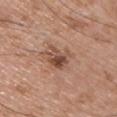The subject is a male aged 48 to 52. The lesion is located on the chest. The lesion's longest dimension is about 3 mm. This image is a 15 mm lesion crop taken from a total-body photograph. The tile uses white-light illumination.